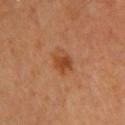This lesion was catalogued during total-body skin photography and was not selected for biopsy. The lesion is on the upper back. Automated image analysis of the tile measured border irregularity of about 2 on a 0–10 scale, a color-variation rating of about 4.5/10, and radial color variation of about 1.5. The analysis additionally found a lesion-detection confidence of about 100/100. Cropped from a whole-body photographic skin survey; the tile spans about 15 mm. Captured under cross-polarized illumination. A female subject, in their mid- to late 30s.